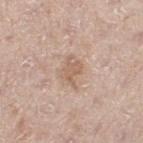Q: Is there a histopathology result?
A: total-body-photography surveillance lesion; no biopsy
Q: What is the imaging modality?
A: 15 mm crop, total-body photography
Q: How was the tile lit?
A: white-light
Q: Lesion size?
A: ~3 mm (longest diameter)
Q: Patient demographics?
A: male, aged 63 to 67
Q: Lesion location?
A: the left thigh